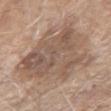  site: right upper arm
  lesion_size:
    long_diameter_mm_approx: 10.0
  image:
    source: total-body photography crop
    field_of_view_mm: 15
  lighting: white-light
  patient:
    sex: female
    age_approx: 85+